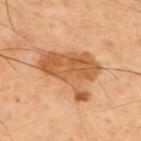Findings:
• follow-up — catalogued during a skin exam; not biopsied
• site — the upper back
• patient — male, in their 60s
• diameter — ≈7.5 mm
• acquisition — 15 mm crop, total-body photography